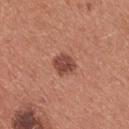Impression: No biopsy was performed on this lesion — it was imaged during a full skin examination and was not determined to be concerning. Acquisition and patient details: On the chest. A 15 mm close-up tile from a total-body photography series done for melanoma screening. The subject is a female aged approximately 35.